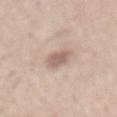Impression:
The lesion was photographed on a routine skin check and not biopsied; there is no pathology result.
Context:
A male patient roughly 40 years of age. Located on the lower back. Longest diameter approximately 2.5 mm. A 15 mm crop from a total-body photograph taken for skin-cancer surveillance.